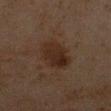<record>
<biopsy_status>not biopsied; imaged during a skin examination</biopsy_status>
<patient>
  <sex>male</sex>
  <age_approx>45</age_approx>
</patient>
<image>
  <source>total-body photography crop</source>
  <field_of_view_mm>15</field_of_view_mm>
</image>
<automated_metrics>
  <area_mm2_approx>12.0</area_mm2_approx>
  <eccentricity>0.65</eccentricity>
  <shape_asymmetry>0.2</shape_asymmetry>
  <cielab_L>20</cielab_L>
  <cielab_a>13</cielab_a>
  <cielab_b>20</cielab_b>
  <vs_skin_darker_L>6.0</vs_skin_darker_L>
  <nevus_likeness_0_100>40</nevus_likeness_0_100>
  <lesion_detection_confidence_0_100>100</lesion_detection_confidence_0_100>
</automated_metrics>
<lesion_size>
  <long_diameter_mm_approx>4.5</long_diameter_mm_approx>
</lesion_size>
<site>arm</site>
<lighting>cross-polarized</lighting>
</record>